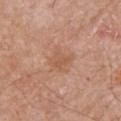Impression:
Captured during whole-body skin photography for melanoma surveillance; the lesion was not biopsied.
Background:
A male patient, aged approximately 80. Imaged with white-light lighting. Cropped from a total-body skin-imaging series; the visible field is about 15 mm. The lesion is located on the arm. Automated image analysis of the tile measured a footprint of about 6.5 mm² and an outline eccentricity of about 0.35 (0 = round, 1 = elongated). The analysis additionally found a border-irregularity index near 3.5/10, internal color variation of about 2 on a 0–10 scale, and peripheral color asymmetry of about 0.5. And it measured a classifier nevus-likeness of about 0/100. The recorded lesion diameter is about 3 mm.